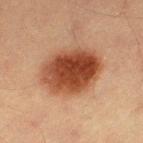Findings:
- lesion size: ~7 mm (longest diameter)
- anatomic site: the left thigh
- subject: male, aged around 40
- imaging modality: total-body-photography crop, ~15 mm field of view